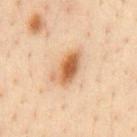biopsy status: catalogued during a skin exam; not biopsied | patient: male, aged approximately 35 | TBP lesion metrics: an average lesion color of about L≈57 a*≈21 b*≈36 (CIELAB), a lesion–skin lightness drop of about 14, and a normalized border contrast of about 9.5; internal color variation of about 6 on a 0–10 scale and peripheral color asymmetry of about 1.5; a lesion-detection confidence of about 100/100 | body site: the chest | diameter: ≈4.5 mm | imaging modality: ~15 mm tile from a whole-body skin photo | lighting: cross-polarized.